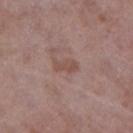notes: imaged on a skin check; not biopsied | patient: female, aged 68 to 72 | acquisition: 15 mm crop, total-body photography | diameter: ~3 mm (longest diameter) | site: the leg.